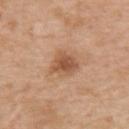<lesion>
<biopsy_status>not biopsied; imaged during a skin examination</biopsy_status>
<image>
  <source>total-body photography crop</source>
  <field_of_view_mm>15</field_of_view_mm>
</image>
<lesion_size>
  <long_diameter_mm_approx>3.5</long_diameter_mm_approx>
</lesion_size>
<patient>
  <sex>male</sex>
  <age_approx>60</age_approx>
</patient>
<automated_metrics>
  <border_irregularity_0_10>3.0</border_irregularity_0_10>
  <color_variation_0_10>3.0</color_variation_0_10>
</automated_metrics>
<site>upper back</site>
<lighting>white-light</lighting>
</lesion>